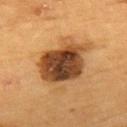workup: total-body-photography surveillance lesion; no biopsy | lighting: cross-polarized illumination | patient: male, in their mid-80s or older | diameter: about 7 mm | body site: the upper back | image-analysis metrics: a lesion area of about 25 mm² and a shape-asymmetry score of about 0.2 (0 = symmetric); a border-irregularity index near 3/10, internal color variation of about 8.5 on a 0–10 scale, and radial color variation of about 2.5; a nevus-likeness score of about 80/100 and lesion-presence confidence of about 100/100 | image source: total-body-photography crop, ~15 mm field of view.